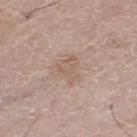Assessment: Imaged during a routine full-body skin examination; the lesion was not biopsied and no histopathology is available. Background: Longest diameter approximately 3.5 mm. This is a white-light tile. A region of skin cropped from a whole-body photographic capture, roughly 15 mm wide. A male patient, about 70 years old. The lesion is on the right leg.